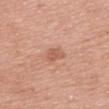workup: total-body-photography surveillance lesion; no biopsy
patient: female, aged 68 to 72
TBP lesion metrics: a lesion color around L≈58 a*≈24 b*≈31 in CIELAB, roughly 9 lightness units darker than nearby skin, and a lesion-to-skin contrast of about 6 (normalized; higher = more distinct); border irregularity of about 3 on a 0–10 scale, internal color variation of about 1 on a 0–10 scale, and peripheral color asymmetry of about 0.5; a nevus-likeness score of about 10/100 and lesion-presence confidence of about 100/100
illumination: white-light illumination
location: the mid back
size: about 3 mm
acquisition: ~15 mm crop, total-body skin-cancer survey